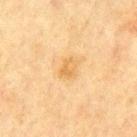biopsy_status: not biopsied; imaged during a skin examination
automated_metrics:
  area_mm2_approx: 4.5
  eccentricity: 0.7
  shape_asymmetry: 0.2
  cielab_L: 58
  cielab_a: 16
  cielab_b: 40
  vs_skin_contrast_norm: 6.0
  border_irregularity_0_10: 2.0
  color_variation_0_10: 3.0
  peripheral_color_asymmetry: 1.0
lesion_size:
  long_diameter_mm_approx: 2.5
lighting: cross-polarized
patient:
  sex: male
  age_approx: 70
image:
  source: total-body photography crop
  field_of_view_mm: 15
site: mid back